This image is a 15 mm lesion crop taken from a total-body photograph. Imaged with white-light lighting. Located on the upper back. The lesion-visualizer software estimated a lesion area of about 1.5 mm², an outline eccentricity of about 0.8 (0 = round, 1 = elongated), and a symmetry-axis asymmetry near 0.6. It also reported a border-irregularity index near 6.5/10, internal color variation of about 0 on a 0–10 scale, and radial color variation of about 0. The software also gave an automated nevus-likeness rating near 0 out of 100 and lesion-presence confidence of about 100/100. A male subject, aged around 65.
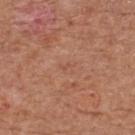On excision, pathology confirmed a nodular basal cell carcinoma.